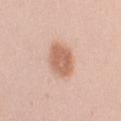biopsy_status: not biopsied; imaged during a skin examination
patient:
  sex: female
  age_approx: 20
automated_metrics:
  nevus_likeness_0_100: 95
  lesion_detection_confidence_0_100: 100
site: left upper arm
lighting: white-light
image:
  source: total-body photography crop
  field_of_view_mm: 15
lesion_size:
  long_diameter_mm_approx: 4.5